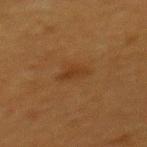Clinical impression: Captured during whole-body skin photography for melanoma surveillance; the lesion was not biopsied.